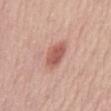– biopsy status — imaged on a skin check; not biopsied
– tile lighting — white-light
– size — ≈3.5 mm
– subject — female, aged 63–67
– anatomic site — the mid back
– TBP lesion metrics — a border-irregularity rating of about 1.5/10, a color-variation rating of about 2.5/10, and radial color variation of about 1; a classifier nevus-likeness of about 95/100 and a lesion-detection confidence of about 100/100
– imaging modality — 15 mm crop, total-body photography